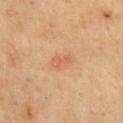Case summary:
– notes — catalogued during a skin exam; not biopsied
– tile lighting — cross-polarized illumination
– image-analysis metrics — a footprint of about 3.5 mm², an outline eccentricity of about 0.85 (0 = round, 1 = elongated), and a symmetry-axis asymmetry near 0.3; a lesion color around L≈58 a*≈23 b*≈35 in CIELAB, a lesion–skin lightness drop of about 6, and a normalized border contrast of about 4.5
– anatomic site — the chest
– image — total-body-photography crop, ~15 mm field of view
– subject — male, roughly 70 years of age